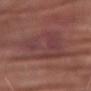Clinical impression:
The lesion was photographed on a routine skin check and not biopsied; there is no pathology result.
Acquisition and patient details:
About 8 mm across. This image is a 15 mm lesion crop taken from a total-body photograph. A male subject about 80 years old. On the left forearm.